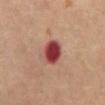biopsy status = imaged on a skin check; not biopsied | imaging modality = ~15 mm tile from a whole-body skin photo | tile lighting = cross-polarized | subject = female, roughly 65 years of age | lesion size = about 3.5 mm | image-analysis metrics = a border-irregularity rating of about 1/10; an automated nevus-likeness rating near 0 out of 100 and a detector confidence of about 100 out of 100 that the crop contains a lesion | body site = the chest.